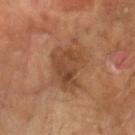Clinical impression:
No biopsy was performed on this lesion — it was imaged during a full skin examination and was not determined to be concerning.
Acquisition and patient details:
Automated tile analysis of the lesion measured a lesion area of about 14 mm², an eccentricity of roughly 0.65, and a symmetry-axis asymmetry near 0.35. The analysis additionally found a border-irregularity index near 4/10, a within-lesion color-variation index near 4.5/10, and peripheral color asymmetry of about 1.5. The software also gave a classifier nevus-likeness of about 25/100 and a lesion-detection confidence of about 100/100. Captured under cross-polarized illumination. A male subject, aged 63 to 67. The recorded lesion diameter is about 5.5 mm. A lesion tile, about 15 mm wide, cut from a 3D total-body photograph. Located on the left forearm.